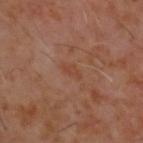Clinical impression:
Imaged during a routine full-body skin examination; the lesion was not biopsied and no histopathology is available.
Clinical summary:
The subject is a male approximately 60 years of age. On the upper back. A roughly 15 mm field-of-view crop from a total-body skin photograph.